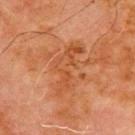Recorded during total-body skin imaging; not selected for excision or biopsy.
A male patient, aged approximately 70.
A 15 mm close-up tile from a total-body photography series done for melanoma screening.
Captured under cross-polarized illumination.
Located on the chest.